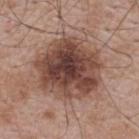  biopsy_status: not biopsied; imaged during a skin examination
  patient:
    sex: male
    age_approx: 65
  lighting: white-light
  lesion_size:
    long_diameter_mm_approx: 7.5
  image:
    source: total-body photography crop
    field_of_view_mm: 15
  site: upper back